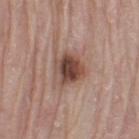Part of a total-body skin-imaging series; this lesion was reviewed on a skin check and was not flagged for biopsy. Cropped from a total-body skin-imaging series; the visible field is about 15 mm. A male subject in their 80s. From the left thigh. Approximately 3.5 mm at its widest. Automated tile analysis of the lesion measured a mean CIELAB color near L≈45 a*≈20 b*≈24 and roughly 14 lightness units darker than nearby skin. The tile uses white-light illumination.